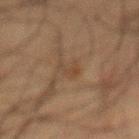{"biopsy_status": "not biopsied; imaged during a skin examination", "lesion_size": {"long_diameter_mm_approx": 2.5}, "automated_metrics": {"area_mm2_approx": 2.5, "eccentricity": 0.8}, "patient": {"sex": "male", "age_approx": 60}, "image": {"source": "total-body photography crop", "field_of_view_mm": 15}, "site": "mid back", "lighting": "cross-polarized"}